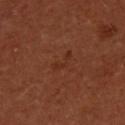biopsy status: catalogued during a skin exam; not biopsied
acquisition: ~15 mm crop, total-body skin-cancer survey
lighting: cross-polarized
subject: female, aged approximately 50
automated lesion analysis: a lesion color around L≈30 a*≈24 b*≈29 in CIELAB, roughly 4 lightness units darker than nearby skin, and a lesion-to-skin contrast of about 5 (normalized; higher = more distinct); a border-irregularity index near 6.5/10, a within-lesion color-variation index near 0/10, and radial color variation of about 0